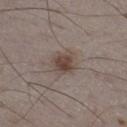No biopsy was performed on this lesion — it was imaged during a full skin examination and was not determined to be concerning. From the leg. A roughly 15 mm field-of-view crop from a total-body skin photograph. The lesion-visualizer software estimated a lesion area of about 7.5 mm², a shape eccentricity near 0.6, and a symmetry-axis asymmetry near 0.2. It also reported a classifier nevus-likeness of about 90/100 and a lesion-detection confidence of about 100/100. A male patient, in their mid-70s. Approximately 3.5 mm at its widest. The tile uses white-light illumination.